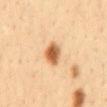Notes:
* biopsy status — total-body-photography surveillance lesion; no biopsy
* image source — 15 mm crop, total-body photography
* patient — male, in their mid-30s
* site — the mid back